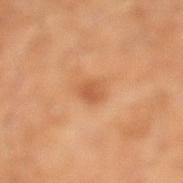follow-up — total-body-photography surveillance lesion; no biopsy | image-analysis metrics — an average lesion color of about L≈55 a*≈26 b*≈39 (CIELAB) and a lesion-to-skin contrast of about 6 (normalized; higher = more distinct); a classifier nevus-likeness of about 10/100 and a detector confidence of about 100 out of 100 that the crop contains a lesion | site — the left lower leg | lighting — cross-polarized illumination | acquisition — total-body-photography crop, ~15 mm field of view | subject — male, in their mid- to late 50s.